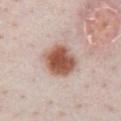image source: 15 mm crop, total-body photography | site: the chest | diameter: about 4.5 mm | patient: male, about 25 years old | lighting: white-light illumination | TBP lesion metrics: a border-irregularity index near 1/10, a color-variation rating of about 6/10, and a peripheral color-asymmetry measure near 1.5; a classifier nevus-likeness of about 100/100 and a lesion-detection confidence of about 100/100.